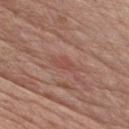Assessment: No biopsy was performed on this lesion — it was imaged during a full skin examination and was not determined to be concerning. Context: The tile uses white-light illumination. The lesion's longest dimension is about 3 mm. Located on the chest. A male patient, approximately 65 years of age. The total-body-photography lesion software estimated an average lesion color of about L≈49 a*≈25 b*≈26 (CIELAB), a lesion–skin lightness drop of about 6, and a lesion-to-skin contrast of about 4.5 (normalized; higher = more distinct). And it measured a border-irregularity rating of about 3.5/10 and a color-variation rating of about 1/10. A 15 mm crop from a total-body photograph taken for skin-cancer surveillance.